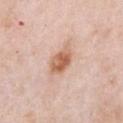Recorded during total-body skin imaging; not selected for excision or biopsy. Cropped from a total-body skin-imaging series; the visible field is about 15 mm. A female subject, in their mid-60s. From the front of the torso. Measured at roughly 4 mm in maximum diameter.